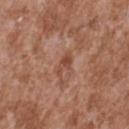workup: catalogued during a skin exam; not biopsied | image: total-body-photography crop, ~15 mm field of view | site: the upper back | subject: male, in their mid-40s | tile lighting: white-light | diameter: about 3 mm.